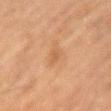Clinical impression:
No biopsy was performed on this lesion — it was imaged during a full skin examination and was not determined to be concerning.
Clinical summary:
The subject is a male roughly 60 years of age. The lesion is located on the left upper arm. A region of skin cropped from a whole-body photographic capture, roughly 15 mm wide. Longest diameter approximately 2.5 mm. This is a cross-polarized tile.